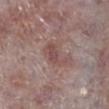Recorded during total-body skin imaging; not selected for excision or biopsy.
The lesion's longest dimension is about 4 mm.
The lesion is on the right lower leg.
A lesion tile, about 15 mm wide, cut from a 3D total-body photograph.
This is a white-light tile.
The subject is a male aged 73–77.